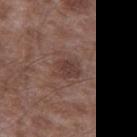No biopsy was performed on this lesion — it was imaged during a full skin examination and was not determined to be concerning. The lesion is on the left thigh. This is a white-light tile. The lesion's longest dimension is about 3 mm. A male patient, aged around 45. Cropped from a total-body skin-imaging series; the visible field is about 15 mm.